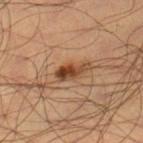<lesion>
  <biopsy_status>not biopsied; imaged during a skin examination</biopsy_status>
  <image>
    <source>total-body photography crop</source>
    <field_of_view_mm>15</field_of_view_mm>
  </image>
  <lighting>cross-polarized</lighting>
  <patient>
    <sex>male</sex>
    <age_approx>35</age_approx>
  </patient>
  <site>right lower leg</site>
  <lesion_size>
    <long_diameter_mm_approx>4.0</long_diameter_mm_approx>
  </lesion_size>
  <automated_metrics>
    <area_mm2_approx>5.5</area_mm2_approx>
    <eccentricity>0.9</eccentricity>
    <shape_asymmetry>0.3</shape_asymmetry>
    <border_irregularity_0_10>3.5</border_irregularity_0_10>
    <color_variation_0_10>4.5</color_variation_0_10>
    <peripheral_color_asymmetry>1.0</peripheral_color_asymmetry>
  </automated_metrics>
</lesion>